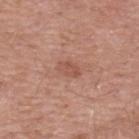Q: Is there a histopathology result?
A: no biopsy performed (imaged during a skin exam)
Q: Automated lesion metrics?
A: an automated nevus-likeness rating near 0 out of 100 and a lesion-detection confidence of about 100/100
Q: Patient demographics?
A: male, aged approximately 55
Q: What kind of image is this?
A: total-body-photography crop, ~15 mm field of view
Q: How large is the lesion?
A: about 2.5 mm
Q: What is the anatomic site?
A: the upper back